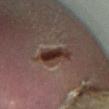Impression:
Captured during whole-body skin photography for melanoma surveillance; the lesion was not biopsied.
Image and clinical context:
This is a cross-polarized tile. About 4.5 mm across. From the left lower leg. A male subject, approximately 65 years of age. Automated tile analysis of the lesion measured a border-irregularity rating of about 3.5/10, a color-variation rating of about 5/10, and radial color variation of about 1.5. And it measured a nevus-likeness score of about 5/100. A close-up tile cropped from a whole-body skin photograph, about 15 mm across.